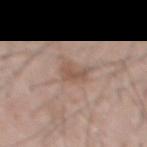Clinical impression:
Imaged during a routine full-body skin examination; the lesion was not biopsied and no histopathology is available.
Acquisition and patient details:
Longest diameter approximately 3 mm. From the abdomen. A male subject aged 53–57. This image is a 15 mm lesion crop taken from a total-body photograph.